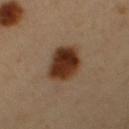No biopsy was performed on this lesion — it was imaged during a full skin examination and was not determined to be concerning. The tile uses cross-polarized illumination. A 15 mm crop from a total-body photograph taken for skin-cancer surveillance. The lesion's longest dimension is about 5 mm. The total-body-photography lesion software estimated a border-irregularity rating of about 1.5/10, a color-variation rating of about 6/10, and peripheral color asymmetry of about 2. It also reported an automated nevus-likeness rating near 100 out of 100 and a lesion-detection confidence of about 100/100. The patient is a male aged approximately 50. On the abdomen.